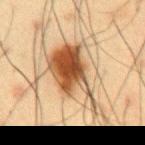Imaged during a routine full-body skin examination; the lesion was not biopsied and no histopathology is available.
This is a cross-polarized tile.
The lesion's longest dimension is about 8 mm.
The lesion is located on the chest.
A roughly 15 mm field-of-view crop from a total-body skin photograph.
The patient is a male aged 58–62.
An algorithmic analysis of the crop reported a mean CIELAB color near L≈43 a*≈17 b*≈30, a lesion–skin lightness drop of about 15, and a lesion-to-skin contrast of about 11.5 (normalized; higher = more distinct). The analysis additionally found a lesion-detection confidence of about 100/100.